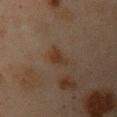Captured during whole-body skin photography for melanoma surveillance; the lesion was not biopsied. A female patient roughly 45 years of age. On the left upper arm. Measured at roughly 2.5 mm in maximum diameter. A 15 mm crop from a total-body photograph taken for skin-cancer surveillance. The total-body-photography lesion software estimated a footprint of about 3 mm². The software also gave a mean CIELAB color near L≈29 a*≈15 b*≈25. It also reported a border-irregularity index near 4/10, internal color variation of about 1.5 on a 0–10 scale, and radial color variation of about 0.5.